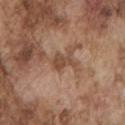{
  "biopsy_status": "not biopsied; imaged during a skin examination",
  "site": "left upper arm",
  "lesion_size": {
    "long_diameter_mm_approx": 3.0
  },
  "lighting": "white-light",
  "automated_metrics": {
    "area_mm2_approx": 4.0,
    "eccentricity": 0.8,
    "shape_asymmetry": 0.45,
    "nevus_likeness_0_100": 0,
    "lesion_detection_confidence_0_100": 100
  },
  "image": {
    "source": "total-body photography crop",
    "field_of_view_mm": 15
  },
  "patient": {
    "sex": "male",
    "age_approx": 75
  }
}